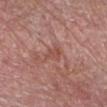Acquisition and patient details:
This is a cross-polarized tile. The lesion-visualizer software estimated a lesion color around L≈43 a*≈21 b*≈23 in CIELAB, roughly 6 lightness units darker than nearby skin, and a lesion-to-skin contrast of about 5.5 (normalized; higher = more distinct). The software also gave a detector confidence of about 95 out of 100 that the crop contains a lesion. The subject is a male approximately 60 years of age. This image is a 15 mm lesion crop taken from a total-body photograph. On the arm.